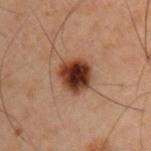biopsy status = no biopsy performed (imaged during a skin exam)
lesion size = ≈3.5 mm
lighting = cross-polarized illumination
TBP lesion metrics = a within-lesion color-variation index near 7.5/10 and peripheral color asymmetry of about 2; a nevus-likeness score of about 100/100 and a detector confidence of about 100 out of 100 that the crop contains a lesion
subject = male, aged approximately 45
image source = 15 mm crop, total-body photography
location = the left upper arm